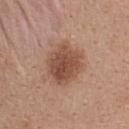Q: Was this lesion biopsied?
A: total-body-photography surveillance lesion; no biopsy
Q: Lesion size?
A: ~5 mm (longest diameter)
Q: Illumination type?
A: white-light
Q: Lesion location?
A: the upper back
Q: How was this image acquired?
A: total-body-photography crop, ~15 mm field of view
Q: Patient demographics?
A: female, approximately 40 years of age
Q: What did automated image analysis measure?
A: a normalized lesion–skin contrast near 8.5; a classifier nevus-likeness of about 85/100 and lesion-presence confidence of about 100/100